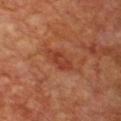This lesion was catalogued during total-body skin photography and was not selected for biopsy.
The lesion-visualizer software estimated a lesion–skin lightness drop of about 7. The analysis additionally found a border-irregularity rating of about 3.5/10, internal color variation of about 3 on a 0–10 scale, and a peripheral color-asymmetry measure near 1. The analysis additionally found a nevus-likeness score of about 0/100.
On the chest.
The recorded lesion diameter is about 4.5 mm.
A close-up tile cropped from a whole-body skin photograph, about 15 mm across.
Captured under cross-polarized illumination.
The patient is a male about 60 years old.